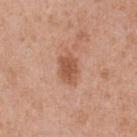follow-up — total-body-photography surveillance lesion; no biopsy
site — the front of the torso
automated metrics — a border-irregularity rating of about 2.5/10, internal color variation of about 2 on a 0–10 scale, and a peripheral color-asymmetry measure near 0.5
subject — male, aged 53 to 57
tile lighting — white-light
imaging modality — 15 mm crop, total-body photography